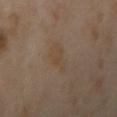Q: Patient demographics?
A: female, aged 38 to 42
Q: What is the anatomic site?
A: the left arm
Q: What is the lesion's diameter?
A: ≈3.5 mm
Q: What kind of image is this?
A: total-body-photography crop, ~15 mm field of view